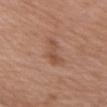Assessment: The lesion was tiled from a total-body skin photograph and was not biopsied. Context: A 15 mm close-up tile from a total-body photography series done for melanoma screening. A female patient, in their 70s. The tile uses white-light illumination. The lesion's longest dimension is about 3.5 mm. Located on the chest.